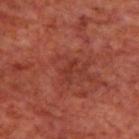follow-up — total-body-photography surveillance lesion; no biopsy
subject — male, in their 70s
lighting — cross-polarized
site — the upper back
acquisition — ~15 mm tile from a whole-body skin photo
size — about 2.5 mm
image-analysis metrics — a footprint of about 2 mm², a shape eccentricity near 0.9, and a shape-asymmetry score of about 0.45 (0 = symmetric); a mean CIELAB color near L≈34 a*≈29 b*≈29, about 5 CIELAB-L* units darker than the surrounding skin, and a lesion-to-skin contrast of about 5 (normalized; higher = more distinct); a classifier nevus-likeness of about 0/100 and a lesion-detection confidence of about 100/100